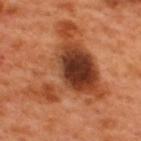follow-up — imaged on a skin check; not biopsied | lighting — cross-polarized | imaging modality — ~15 mm tile from a whole-body skin photo | patient — male, approximately 50 years of age | site — the upper back.